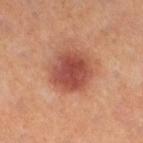Impression: Recorded during total-body skin imaging; not selected for excision or biopsy. Background: A female patient, approximately 55 years of age. A 15 mm crop from a total-body photograph taken for skin-cancer surveillance. Located on the right thigh.